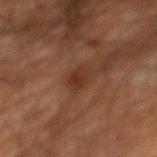| key | value |
|---|---|
| biopsy status | catalogued during a skin exam; not biopsied |
| anatomic site | the mid back |
| lighting | cross-polarized |
| patient | male, aged 58–62 |
| TBP lesion metrics | a footprint of about 3.5 mm², an outline eccentricity of about 0.75 (0 = round, 1 = elongated), and a shape-asymmetry score of about 0.2 (0 = symmetric); a lesion color around L≈24 a*≈17 b*≈23 in CIELAB, about 6 CIELAB-L* units darker than the surrounding skin, and a lesion-to-skin contrast of about 7 (normalized; higher = more distinct) |
| imaging modality | total-body-photography crop, ~15 mm field of view |
| diameter | ≈2.5 mm |